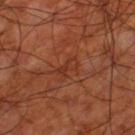<record>
<biopsy_status>not biopsied; imaged during a skin examination</biopsy_status>
<patient>
  <sex>male</sex>
  <age_approx>70</age_approx>
</patient>
<automated_metrics>
  <area_mm2_approx>3.5</area_mm2_approx>
  <eccentricity>0.85</eccentricity>
  <shape_asymmetry>0.3</shape_asymmetry>
  <cielab_L>33</cielab_L>
  <cielab_a>26</cielab_a>
  <cielab_b>30</cielab_b>
  <vs_skin_darker_L>6.0</vs_skin_darker_L>
  <nevus_likeness_0_100>0</nevus_likeness_0_100>
  <lesion_detection_confidence_0_100>95</lesion_detection_confidence_0_100>
</automated_metrics>
<site>leg</site>
<lighting>cross-polarized</lighting>
<image>
  <source>total-body photography crop</source>
  <field_of_view_mm>15</field_of_view_mm>
</image>
</record>